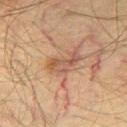Captured during whole-body skin photography for melanoma surveillance; the lesion was not biopsied.
A male patient, approximately 50 years of age.
Approximately 4 mm at its widest.
Imaged with cross-polarized lighting.
From the right thigh.
Cropped from a total-body skin-imaging series; the visible field is about 15 mm.
The total-body-photography lesion software estimated a lesion area of about 6 mm² and a shape eccentricity near 0.9. The analysis additionally found a lesion color around L≈43 a*≈18 b*≈26 in CIELAB and a lesion–skin lightness drop of about 8. And it measured a border-irregularity index near 5/10, a color-variation rating of about 3.5/10, and a peripheral color-asymmetry measure near 0.5.